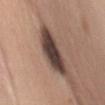Assessment: Recorded during total-body skin imaging; not selected for excision or biopsy. Clinical summary: A female subject aged 48 to 52. A 15 mm close-up tile from a total-body photography series done for melanoma screening. The lesion is on the chest.